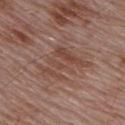| key | value |
|---|---|
| notes | catalogued during a skin exam; not biopsied |
| diameter | about 6 mm |
| patient | male, in their mid- to late 50s |
| body site | the upper back |
| image | ~15 mm tile from a whole-body skin photo |
| lighting | white-light illumination |